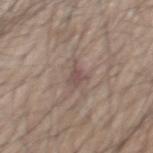Part of a total-body skin-imaging series; this lesion was reviewed on a skin check and was not flagged for biopsy. The lesion is located on the mid back. Cropped from a total-body skin-imaging series; the visible field is about 15 mm. The patient is a male roughly 70 years of age. This is a white-light tile. An algorithmic analysis of the crop reported an average lesion color of about L≈49 a*≈15 b*≈20 (CIELAB), a lesion–skin lightness drop of about 8, and a normalized lesion–skin contrast near 6.5. It also reported border irregularity of about 5 on a 0–10 scale, a color-variation rating of about 2/10, and a peripheral color-asymmetry measure near 0.5.